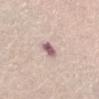<record>
<biopsy_status>not biopsied; imaged during a skin examination</biopsy_status>
<lighting>white-light</lighting>
<image>
  <source>total-body photography crop</source>
  <field_of_view_mm>15</field_of_view_mm>
</image>
<automated_metrics>
  <cielab_L>59</cielab_L>
  <cielab_a>21</cielab_a>
  <cielab_b>17</cielab_b>
  <vs_skin_darker_L>16.0</vs_skin_darker_L>
  <vs_skin_contrast_norm>10.5</vs_skin_contrast_norm>
  <border_irregularity_0_10>2.0</border_irregularity_0_10>
  <color_variation_0_10>2.5</color_variation_0_10>
</automated_metrics>
<site>abdomen</site>
<patient>
  <sex>female</sex>
  <age_approx>65</age_approx>
</patient>
</record>